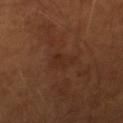This lesion was catalogued during total-body skin photography and was not selected for biopsy. About 3 mm across. Imaged with cross-polarized lighting. Cropped from a whole-body photographic skin survey; the tile spans about 15 mm. A male subject, aged 63–67.